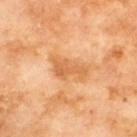No biopsy was performed on this lesion — it was imaged during a full skin examination and was not determined to be concerning. A male subject approximately 70 years of age. On the upper back. A roughly 15 mm field-of-view crop from a total-body skin photograph.